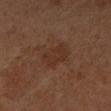The lesion was tiled from a total-body skin photograph and was not biopsied. This is a cross-polarized tile. Measured at roughly 3.5 mm in maximum diameter. A lesion tile, about 15 mm wide, cut from a 3D total-body photograph. The lesion is on the arm. Automated tile analysis of the lesion measured a mean CIELAB color near L≈27 a*≈17 b*≈23, a lesion–skin lightness drop of about 5, and a normalized border contrast of about 5.5. The analysis additionally found a border-irregularity index near 2.5/10, a color-variation rating of about 1.5/10, and radial color variation of about 0.5. It also reported a nevus-likeness score of about 0/100. The patient is a female in their 60s.